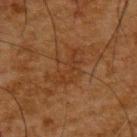The lesion was photographed on a routine skin check and not biopsied; there is no pathology result.
Automated image analysis of the tile measured an eccentricity of roughly 0.8 and two-axis asymmetry of about 0.45. The analysis additionally found a lesion color around L≈30 a*≈18 b*≈29 in CIELAB, roughly 4 lightness units darker than nearby skin, and a normalized border contrast of about 4.5. It also reported a border-irregularity index near 7/10, internal color variation of about 3 on a 0–10 scale, and peripheral color asymmetry of about 1.
This image is a 15 mm lesion crop taken from a total-body photograph.
The lesion is located on the upper back.
Imaged with cross-polarized lighting.
A male subject aged around 65.
The recorded lesion diameter is about 5.5 mm.